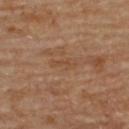Imaged during a routine full-body skin examination; the lesion was not biopsied and no histopathology is available. The tile uses cross-polarized illumination. The total-body-photography lesion software estimated a lesion area of about 2 mm², a shape eccentricity near 0.95, and a shape-asymmetry score of about 0.35 (0 = symmetric). And it measured a border-irregularity rating of about 4.5/10, a within-lesion color-variation index near 0/10, and radial color variation of about 0. And it measured a nevus-likeness score of about 0/100. Longest diameter approximately 3 mm. From the upper back. Cropped from a total-body skin-imaging series; the visible field is about 15 mm. A male subject, aged approximately 85 or older.